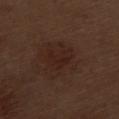biopsy status = no biopsy performed (imaged during a skin exam) | imaging modality = total-body-photography crop, ~15 mm field of view | diameter = about 3 mm | lighting = white-light illumination | site = the leg | patient = male, approximately 70 years of age.